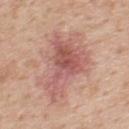Recorded during total-body skin imaging; not selected for excision or biopsy. A lesion tile, about 15 mm wide, cut from a 3D total-body photograph. On the upper back. A male subject aged approximately 50.